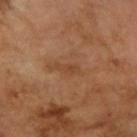A female patient approximately 70 years of age.
On the arm.
Cropped from a total-body skin-imaging series; the visible field is about 15 mm.